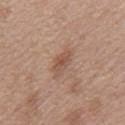Recorded during total-body skin imaging; not selected for excision or biopsy. Located on the mid back. An algorithmic analysis of the crop reported a shape eccentricity near 0.9 and a symmetry-axis asymmetry near 0.25. The analysis additionally found a border-irregularity rating of about 3/10. The subject is a female aged around 75. A lesion tile, about 15 mm wide, cut from a 3D total-body photograph. About 4 mm across.